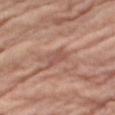Case summary:
• biopsy status: imaged on a skin check; not biopsied
• image source: total-body-photography crop, ~15 mm field of view
• TBP lesion metrics: an average lesion color of about L≈53 a*≈22 b*≈26 (CIELAB), about 8 CIELAB-L* units darker than the surrounding skin, and a lesion-to-skin contrast of about 5.5 (normalized; higher = more distinct); a border-irregularity rating of about 4/10 and a color-variation rating of about 2.5/10
• anatomic site: the right thigh
• tile lighting: white-light illumination
• patient: female, in their mid- to late 70s
• lesion diameter: ~3 mm (longest diameter)